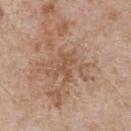About 3 mm across.
This image is a 15 mm lesion crop taken from a total-body photograph.
Imaged with white-light lighting.
A male patient in their mid-60s.
The total-body-photography lesion software estimated a border-irregularity rating of about 6/10, internal color variation of about 1 on a 0–10 scale, and peripheral color asymmetry of about 0.5.
The lesion is on the chest.